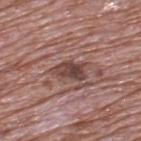follow-up — imaged on a skin check; not biopsied | tile lighting — white-light | imaging modality — 15 mm crop, total-body photography | patient — male, aged 68 to 72 | size — about 3.5 mm | location — the upper back.